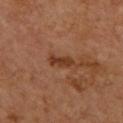{
  "biopsy_status": "not biopsied; imaged during a skin examination"
}